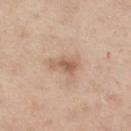illumination = white-light illumination
body site = the right thigh
automated metrics = a border-irregularity index near 4/10, a within-lesion color-variation index near 2.5/10, and radial color variation of about 1; a nevus-likeness score of about 25/100 and a detector confidence of about 100 out of 100 that the crop contains a lesion
image source = ~15 mm tile from a whole-body skin photo
lesion diameter = about 3.5 mm
patient = female, about 55 years old